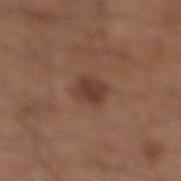This lesion was catalogued during total-body skin photography and was not selected for biopsy. The subject is a male aged around 60. A region of skin cropped from a whole-body photographic capture, roughly 15 mm wide. The lesion is on the leg.